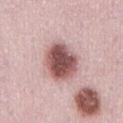biopsy status — imaged on a skin check; not biopsied
site — the abdomen
subject — female, aged around 30
image source — 15 mm crop, total-body photography